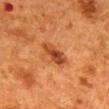Imaged during a routine full-body skin examination; the lesion was not biopsied and no histopathology is available. The lesion is located on the mid back. This is a cross-polarized tile. Longest diameter approximately 4 mm. A lesion tile, about 15 mm wide, cut from a 3D total-body photograph. The subject is a female aged 48 to 52. An algorithmic analysis of the crop reported a color-variation rating of about 4/10 and peripheral color asymmetry of about 1.5.